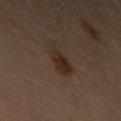No biopsy was performed on this lesion — it was imaged during a full skin examination and was not determined to be concerning. The tile uses cross-polarized illumination. A close-up tile cropped from a whole-body skin photograph, about 15 mm across. The lesion is on the left upper arm. A female subject in their 30s. The lesion's longest dimension is about 3.5 mm. Automated image analysis of the tile measured a mean CIELAB color near L≈20 a*≈11 b*≈18, a lesion–skin lightness drop of about 5, and a normalized lesion–skin contrast near 7.5. It also reported a border-irregularity rating of about 3.5/10 and peripheral color asymmetry of about 1. The software also gave a nevus-likeness score of about 75/100 and a lesion-detection confidence of about 100/100.